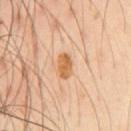Assessment: The lesion was photographed on a routine skin check and not biopsied; there is no pathology result. Context: Longest diameter approximately 3 mm. A region of skin cropped from a whole-body photographic capture, roughly 15 mm wide. The tile uses cross-polarized illumination. A male subject, in their mid- to late 40s. The total-body-photography lesion software estimated a footprint of about 5 mm² and a shape eccentricity near 0.8. It also reported border irregularity of about 1.5 on a 0–10 scale, internal color variation of about 3.5 on a 0–10 scale, and a peripheral color-asymmetry measure near 1.5. The analysis additionally found an automated nevus-likeness rating near 75 out of 100. From the front of the torso.